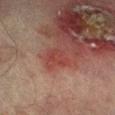Captured during whole-body skin photography for melanoma surveillance; the lesion was not biopsied. A male patient, in their mid-70s. About 3 mm across. The lesion is located on the leg. Captured under cross-polarized illumination. A 15 mm close-up tile from a total-body photography series done for melanoma screening.